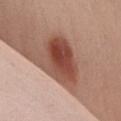The lesion was tiled from a total-body skin photograph and was not biopsied. A female patient in their mid- to late 20s. On the chest. A roughly 15 mm field-of-view crop from a total-body skin photograph. Imaged with white-light lighting. The lesion's longest dimension is about 7 mm. The total-body-photography lesion software estimated a border-irregularity index near 2.5/10, internal color variation of about 8 on a 0–10 scale, and a peripheral color-asymmetry measure near 2.5. It also reported an automated nevus-likeness rating near 95 out of 100 and a lesion-detection confidence of about 100/100.